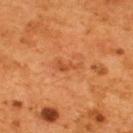The lesion was tiled from a total-body skin photograph and was not biopsied. A region of skin cropped from a whole-body photographic capture, roughly 15 mm wide. On the upper back. The lesion-visualizer software estimated a footprint of about 3 mm² and a symmetry-axis asymmetry near 0.5. The analysis additionally found a border-irregularity index near 5.5/10 and radial color variation of about 0. And it measured lesion-presence confidence of about 100/100. The recorded lesion diameter is about 3 mm. Captured under cross-polarized illumination. A male patient, aged 53–57.